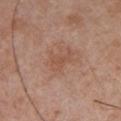Q: Was a biopsy performed?
A: no biopsy performed (imaged during a skin exam)
Q: Where on the body is the lesion?
A: the chest
Q: Illumination type?
A: white-light
Q: Automated lesion metrics?
A: a classifier nevus-likeness of about 0/100 and lesion-presence confidence of about 100/100
Q: Patient demographics?
A: male, aged 28–32
Q: How was this image acquired?
A: 15 mm crop, total-body photography
Q: How large is the lesion?
A: ~3 mm (longest diameter)